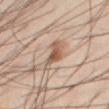Clinical impression: Captured during whole-body skin photography for melanoma surveillance; the lesion was not biopsied. Context: A roughly 15 mm field-of-view crop from a total-body skin photograph. The lesion is on the right thigh. A male subject, in their 40s.